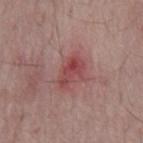{
  "biopsy_status": "not biopsied; imaged during a skin examination",
  "image": {
    "source": "total-body photography crop",
    "field_of_view_mm": 15
  },
  "site": "mid back",
  "patient": {
    "sex": "male",
    "age_approx": 65
  }
}